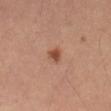Acquisition and patient details:
A male subject, about 50 years old. Cropped from a whole-body photographic skin survey; the tile spans about 15 mm. The lesion is located on the mid back.